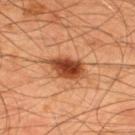The lesion is located on the upper back. A male subject, aged approximately 45. A close-up tile cropped from a whole-body skin photograph, about 15 mm across.